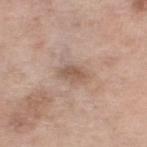Assessment: No biopsy was performed on this lesion — it was imaged during a full skin examination and was not determined to be concerning. Acquisition and patient details: On the left lower leg. An algorithmic analysis of the crop reported an outline eccentricity of about 0.8 (0 = round, 1 = elongated) and a shape-asymmetry score of about 0.25 (0 = symmetric). About 3 mm across. A female patient, aged approximately 55. Imaged with white-light lighting. A roughly 15 mm field-of-view crop from a total-body skin photograph.